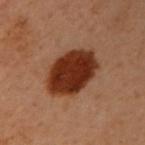Imaged during a routine full-body skin examination; the lesion was not biopsied and no histopathology is available. A roughly 15 mm field-of-view crop from a total-body skin photograph. The lesion is located on the left arm. The patient is a female about 60 years old. Captured under cross-polarized illumination. Longest diameter approximately 6.5 mm.